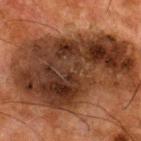{
  "biopsy_status": "not biopsied; imaged during a skin examination",
  "patient": {
    "sex": "male",
    "age_approx": 65
  },
  "lesion_size": {
    "long_diameter_mm_approx": 14.5
  },
  "automated_metrics": {
    "cielab_L": 29,
    "cielab_a": 18,
    "cielab_b": 26,
    "vs_skin_darker_L": 13.0,
    "vs_skin_contrast_norm": 12.0,
    "nevus_likeness_0_100": 0,
    "lesion_detection_confidence_0_100": 100
  },
  "lighting": "cross-polarized",
  "site": "upper back",
  "image": {
    "source": "total-body photography crop",
    "field_of_view_mm": 15
  }
}